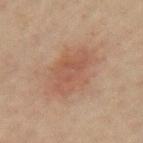<case>
  <biopsy_status>not biopsied; imaged during a skin examination</biopsy_status>
  <automated_metrics>
    <area_mm2_approx>17.0</area_mm2_approx>
    <eccentricity>0.8</eccentricity>
    <shape_asymmetry>0.25</shape_asymmetry>
    <border_irregularity_0_10>3.5</border_irregularity_0_10>
    <color_variation_0_10>2.5</color_variation_0_10>
    <nevus_likeness_0_100>60</nevus_likeness_0_100>
    <lesion_detection_confidence_0_100>100</lesion_detection_confidence_0_100>
  </automated_metrics>
  <image>
    <source>total-body photography crop</source>
    <field_of_view_mm>15</field_of_view_mm>
  </image>
  <site>left upper arm</site>
  <patient>
    <sex>female</sex>
    <age_approx>45</age_approx>
  </patient>
</case>